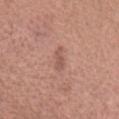Imaged during a routine full-body skin examination; the lesion was not biopsied and no histopathology is available. A female subject, aged 38–42. Automated image analysis of the tile measured border irregularity of about 4.5 on a 0–10 scale, internal color variation of about 0 on a 0–10 scale, and peripheral color asymmetry of about 0. This image is a 15 mm lesion crop taken from a total-body photograph. Imaged with white-light lighting. The lesion is on the head or neck. The recorded lesion diameter is about 3 mm.